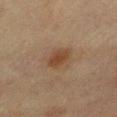Captured during whole-body skin photography for melanoma surveillance; the lesion was not biopsied. The lesion is located on the front of the torso. The lesion-visualizer software estimated an eccentricity of roughly 0.75 and two-axis asymmetry of about 0.2. And it measured a lesion color around L≈37 a*≈16 b*≈27 in CIELAB and a normalized border contrast of about 7.5. And it measured an automated nevus-likeness rating near 90 out of 100 and lesion-presence confidence of about 100/100. The tile uses cross-polarized illumination. A female subject, aged approximately 65. A roughly 15 mm field-of-view crop from a total-body skin photograph. Measured at roughly 3.5 mm in maximum diameter.